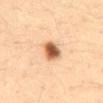Impression:
The lesion was tiled from a total-body skin photograph and was not biopsied.
Clinical summary:
Imaged with cross-polarized lighting. An algorithmic analysis of the crop reported a footprint of about 6 mm² and a symmetry-axis asymmetry near 0.25. The analysis additionally found a mean CIELAB color near L≈55 a*≈22 b*≈34, about 19 CIELAB-L* units darker than the surrounding skin, and a lesion-to-skin contrast of about 12 (normalized; higher = more distinct). It also reported radial color variation of about 1.5. A close-up tile cropped from a whole-body skin photograph, about 15 mm across. A male patient approximately 35 years of age. Located on the back. Measured at roughly 3 mm in maximum diameter.